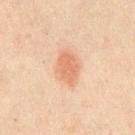Q: Is there a histopathology result?
A: catalogued during a skin exam; not biopsied
Q: Automated lesion metrics?
A: a footprint of about 8 mm² and an outline eccentricity of about 0.75 (0 = round, 1 = elongated); an average lesion color of about L≈58 a*≈21 b*≈31 (CIELAB), roughly 9 lightness units darker than nearby skin, and a lesion-to-skin contrast of about 6.5 (normalized; higher = more distinct); an automated nevus-likeness rating near 100 out of 100 and lesion-presence confidence of about 100/100
Q: Who is the patient?
A: male, roughly 45 years of age
Q: What kind of image is this?
A: ~15 mm crop, total-body skin-cancer survey
Q: What is the anatomic site?
A: the chest
Q: How large is the lesion?
A: ~3.5 mm (longest diameter)